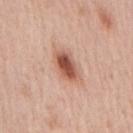Q: Was this lesion biopsied?
A: no biopsy performed (imaged during a skin exam)
Q: Who is the patient?
A: male, aged 63 to 67
Q: Where on the body is the lesion?
A: the front of the torso
Q: How was this image acquired?
A: total-body-photography crop, ~15 mm field of view